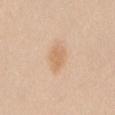notes = imaged on a skin check; not biopsied
lighting = white-light
anatomic site = the mid back
diameter = about 3.5 mm
automated lesion analysis = roughly 8 lightness units darker than nearby skin and a normalized lesion–skin contrast near 6; a border-irregularity index near 2/10 and a within-lesion color-variation index near 2/10
imaging modality = ~15 mm tile from a whole-body skin photo
patient = female, approximately 40 years of age